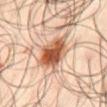An algorithmic analysis of the crop reported a footprint of about 13 mm² and an eccentricity of roughly 0.6.
A male patient in their mid- to late 60s.
The tile uses cross-polarized illumination.
The recorded lesion diameter is about 4.5 mm.
A 15 mm crop from a total-body photograph taken for skin-cancer surveillance.
The lesion is located on the abdomen.